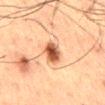{
  "lighting": "cross-polarized",
  "automated_metrics": {
    "area_mm2_approx": 6.0,
    "eccentricity": 0.8,
    "border_irregularity_0_10": 2.5,
    "color_variation_0_10": 5.5,
    "peripheral_color_asymmetry": 1.5,
    "nevus_likeness_0_100": 100
  },
  "image": {
    "source": "total-body photography crop",
    "field_of_view_mm": 15
  },
  "lesion_size": {
    "long_diameter_mm_approx": 3.5
  },
  "patient": {
    "sex": "male",
    "age_approx": 60
  },
  "site": "back"
}